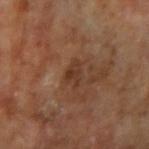The lesion was tiled from a total-body skin photograph and was not biopsied. Measured at roughly 3 mm in maximum diameter. A male subject roughly 65 years of age. The lesion is located on the right upper arm. A 15 mm close-up tile from a total-body photography series done for melanoma screening. Automated image analysis of the tile measured a lesion area of about 4 mm², a shape eccentricity near 0.75, and two-axis asymmetry of about 0.4. It also reported an average lesion color of about L≈34 a*≈19 b*≈27 (CIELAB), about 7 CIELAB-L* units darker than the surrounding skin, and a normalized lesion–skin contrast near 7. It also reported a border-irregularity index near 4/10 and a color-variation rating of about 2.5/10. And it measured an automated nevus-likeness rating near 0 out of 100 and lesion-presence confidence of about 100/100. This is a cross-polarized tile.